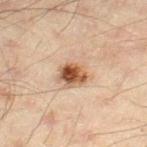Assessment:
Captured during whole-body skin photography for melanoma surveillance; the lesion was not biopsied.
Clinical summary:
Located on the leg. The patient is a male about 45 years old. The tile uses cross-polarized illumination. A close-up tile cropped from a whole-body skin photograph, about 15 mm across. The lesion-visualizer software estimated an area of roughly 11 mm². The analysis additionally found a lesion color around L≈51 a*≈17 b*≈29 in CIELAB, roughly 12 lightness units darker than nearby skin, and a lesion-to-skin contrast of about 8.5 (normalized; higher = more distinct). And it measured a border-irregularity rating of about 3/10.